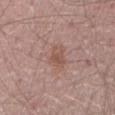Imaged during a routine full-body skin examination; the lesion was not biopsied and no histopathology is available.
This is a white-light tile.
Located on the mid back.
A male subject, about 65 years old.
About 3 mm across.
A 15 mm close-up extracted from a 3D total-body photography capture.